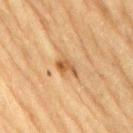Clinical impression:
Captured during whole-body skin photography for melanoma surveillance; the lesion was not biopsied.
Background:
The lesion is on the lower back. Automated image analysis of the tile measured an area of roughly 3.5 mm² and an outline eccentricity of about 0.8 (0 = round, 1 = elongated). The tile uses cross-polarized illumination. A 15 mm close-up extracted from a 3D total-body photography capture. A male subject aged around 85 or older.